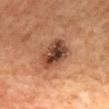Imaged during a routine full-body skin examination; the lesion was not biopsied and no histopathology is available. A lesion tile, about 15 mm wide, cut from a 3D total-body photograph. A female subject, aged approximately 60. On the mid back. The tile uses cross-polarized illumination.